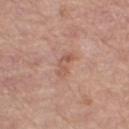Assessment: This lesion was catalogued during total-body skin photography and was not selected for biopsy. Clinical summary: The tile uses white-light illumination. Automated image analysis of the tile measured an area of roughly 4 mm². The analysis additionally found about 8 CIELAB-L* units darker than the surrounding skin and a normalized border contrast of about 5.5. The software also gave a border-irregularity index near 4/10, a color-variation rating of about 2/10, and radial color variation of about 0.5. And it measured a nevus-likeness score of about 0/100 and a detector confidence of about 100 out of 100 that the crop contains a lesion. The recorded lesion diameter is about 3 mm. A female patient, aged around 55. From the right thigh. A 15 mm crop from a total-body photograph taken for skin-cancer surveillance.